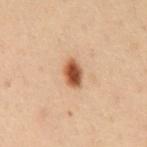No biopsy was performed on this lesion — it was imaged during a full skin examination and was not determined to be concerning.
The patient is a female aged 48 to 52.
A 15 mm close-up extracted from a 3D total-body photography capture.
This is a cross-polarized tile.
The total-body-photography lesion software estimated a lesion area of about 5.5 mm², an outline eccentricity of about 0.75 (0 = round, 1 = elongated), and a symmetry-axis asymmetry near 0.15. It also reported border irregularity of about 1.5 on a 0–10 scale and a color-variation rating of about 4.5/10. The analysis additionally found a nevus-likeness score of about 100/100.
About 3 mm across.
The lesion is on the mid back.